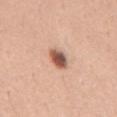No biopsy was performed on this lesion — it was imaged during a full skin examination and was not determined to be concerning. A male patient, about 40 years old. Captured under white-light illumination. From the chest. Automated tile analysis of the lesion measured an average lesion color of about L≈55 a*≈23 b*≈29 (CIELAB), roughly 18 lightness units darker than nearby skin, and a normalized border contrast of about 11. And it measured a color-variation rating of about 5/10 and peripheral color asymmetry of about 1.5. The software also gave a detector confidence of about 100 out of 100 that the crop contains a lesion. This image is a 15 mm lesion crop taken from a total-body photograph. The lesion's longest dimension is about 3 mm.